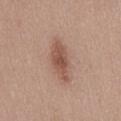Impression:
Part of a total-body skin-imaging series; this lesion was reviewed on a skin check and was not flagged for biopsy.
Background:
The lesion is located on the abdomen. A male patient aged 48 to 52. A region of skin cropped from a whole-body photographic capture, roughly 15 mm wide. Imaged with white-light lighting.